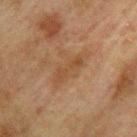The lesion was tiled from a total-body skin photograph and was not biopsied.
The patient is a male about 75 years old.
This image is a 15 mm lesion crop taken from a total-body photograph.
On the left upper arm.